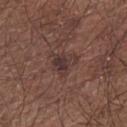Imaged during a routine full-body skin examination; the lesion was not biopsied and no histopathology is available.
On the left upper arm.
The lesion-visualizer software estimated border irregularity of about 5 on a 0–10 scale, a within-lesion color-variation index near 4/10, and peripheral color asymmetry of about 1.
A male patient, aged 63–67.
The tile uses white-light illumination.
A 15 mm crop from a total-body photograph taken for skin-cancer surveillance.
The lesion's longest dimension is about 3.5 mm.